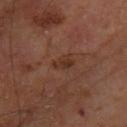workup = catalogued during a skin exam; not biopsied | size = about 3 mm | lighting = cross-polarized illumination | image source = 15 mm crop, total-body photography | subject = male, about 65 years old | site = the upper back.